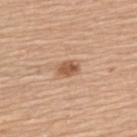{"biopsy_status": "not biopsied; imaged during a skin examination", "automated_metrics": {"area_mm2_approx": 4.5, "eccentricity": 0.75, "shape_asymmetry": 0.25, "nevus_likeness_0_100": 80, "lesion_detection_confidence_0_100": 100}, "image": {"source": "total-body photography crop", "field_of_view_mm": 15}, "lighting": "white-light", "patient": {"sex": "female", "age_approx": 60}, "site": "upper back"}